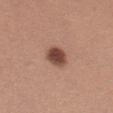The lesion was tiled from a total-body skin photograph and was not biopsied.
A female subject aged 38 to 42.
Captured under white-light illumination.
A lesion tile, about 15 mm wide, cut from a 3D total-body photograph.
The recorded lesion diameter is about 3 mm.
Located on the left thigh.